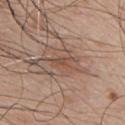follow-up=total-body-photography surveillance lesion; no biopsy
image-analysis metrics=a mean CIELAB color near L≈49 a*≈19 b*≈27, roughly 7 lightness units darker than nearby skin, and a normalized border contrast of about 5.5; border irregularity of about 4 on a 0–10 scale and radial color variation of about 0
lesion diameter=about 3 mm
anatomic site=the chest
acquisition=total-body-photography crop, ~15 mm field of view
subject=male, aged 48 to 52